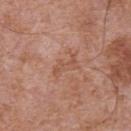* body site · the chest
* diameter · ≈3.5 mm
* image · ~15 mm crop, total-body skin-cancer survey
* lighting · white-light
* patient · male, approximately 70 years of age